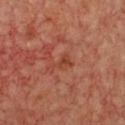Q: How was the tile lit?
A: cross-polarized
Q: Where on the body is the lesion?
A: the chest
Q: Who is the patient?
A: female, aged around 45
Q: What is the lesion's diameter?
A: ≈2.5 mm
Q: How was this image acquired?
A: ~15 mm crop, total-body skin-cancer survey